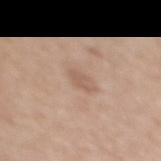| field | value |
|---|---|
| biopsy status | total-body-photography surveillance lesion; no biopsy |
| lighting | white-light illumination |
| location | the upper back |
| subject | female, aged 58 to 62 |
| image | ~15 mm tile from a whole-body skin photo |
| automated metrics | a lesion area of about 2.5 mm², a shape eccentricity near 0.85, and two-axis asymmetry of about 0.35; a border-irregularity rating of about 3.5/10, a color-variation rating of about 0/10, and a peripheral color-asymmetry measure near 0 |
| lesion diameter | ≈2.5 mm |